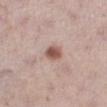Clinical impression: This lesion was catalogued during total-body skin photography and was not selected for biopsy. Context: The tile uses white-light illumination. On the left lower leg. A 15 mm close-up tile from a total-body photography series done for melanoma screening. The recorded lesion diameter is about 2.5 mm. The patient is a female aged approximately 40.